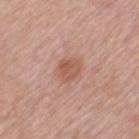<record>
<biopsy_status>not biopsied; imaged during a skin examination</biopsy_status>
<lesion_size>
  <long_diameter_mm_approx>2.5</long_diameter_mm_approx>
</lesion_size>
<image>
  <source>total-body photography crop</source>
  <field_of_view_mm>15</field_of_view_mm>
</image>
<site>upper back</site>
<patient>
  <sex>male</sex>
  <age_approx>55</age_approx>
</patient>
</record>